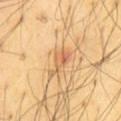Imaged during a routine full-body skin examination; the lesion was not biopsied and no histopathology is available. An algorithmic analysis of the crop reported an average lesion color of about L≈65 a*≈22 b*≈41 (CIELAB) and a lesion-to-skin contrast of about 6 (normalized; higher = more distinct). The software also gave a border-irregularity index near 5.5/10 and internal color variation of about 4.5 on a 0–10 scale. The software also gave a nevus-likeness score of about 20/100. The recorded lesion diameter is about 4 mm. The lesion is on the abdomen. A 15 mm close-up extracted from a 3D total-body photography capture. A male subject aged around 65.